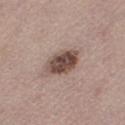The lesion was tiled from a total-body skin photograph and was not biopsied. A female subject, aged 48–52. Longest diameter approximately 4 mm. Cropped from a total-body skin-imaging series; the visible field is about 15 mm. Located on the left thigh. This is a white-light tile.